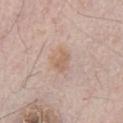Case summary:
- tile lighting — white-light
- patient — male, in their mid-60s
- imaging modality — 15 mm crop, total-body photography
- lesion diameter — about 3 mm
- automated metrics — a footprint of about 5 mm², a shape eccentricity near 0.65, and a shape-asymmetry score of about 0.3 (0 = symmetric); a lesion color around L≈62 a*≈17 b*≈28 in CIELAB, roughly 7 lightness units darker than nearby skin, and a normalized border contrast of about 5.5; border irregularity of about 3 on a 0–10 scale, a color-variation rating of about 2.5/10, and a peripheral color-asymmetry measure near 1
- anatomic site — the front of the torso